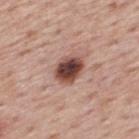{
  "biopsy_status": "not biopsied; imaged during a skin examination",
  "automated_metrics": {
    "cielab_L": 45,
    "cielab_a": 22,
    "cielab_b": 24,
    "vs_skin_darker_L": 19.0,
    "vs_skin_contrast_norm": 13.5,
    "nevus_likeness_0_100": 95,
    "lesion_detection_confidence_0_100": 100
  },
  "patient": {
    "sex": "male",
    "age_approx": 75
  },
  "site": "upper back",
  "lesion_size": {
    "long_diameter_mm_approx": 3.5
  },
  "image": {
    "source": "total-body photography crop",
    "field_of_view_mm": 15
  }
}